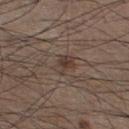Q: Was this lesion biopsied?
A: catalogued during a skin exam; not biopsied
Q: What kind of image is this?
A: ~15 mm crop, total-body skin-cancer survey
Q: Lesion location?
A: the left lower leg
Q: Lesion size?
A: ≈3 mm
Q: Patient demographics?
A: male, in their mid- to late 30s